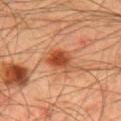Captured during whole-body skin photography for melanoma surveillance; the lesion was not biopsied. On the mid back. A male subject aged around 45. Captured under cross-polarized illumination. Longest diameter approximately 3.5 mm. A lesion tile, about 15 mm wide, cut from a 3D total-body photograph.